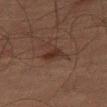follow-up = catalogued during a skin exam; not biopsied
image source = total-body-photography crop, ~15 mm field of view
site = the left thigh
TBP lesion metrics = a mean CIELAB color near L≈25 a*≈15 b*≈20 and a lesion–skin lightness drop of about 5; a border-irregularity index near 5.5/10
diameter = ≈4 mm
lighting = cross-polarized illumination
patient = male, about 65 years old